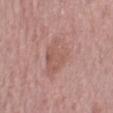The lesion was photographed on a routine skin check and not biopsied; there is no pathology result.
The subject is a female approximately 60 years of age.
The lesion is located on the left lower leg.
An algorithmic analysis of the crop reported a footprint of about 14 mm², an eccentricity of roughly 0.65, and two-axis asymmetry of about 0.3. The software also gave about 7 CIELAB-L* units darker than the surrounding skin and a lesion-to-skin contrast of about 5 (normalized; higher = more distinct).
A lesion tile, about 15 mm wide, cut from a 3D total-body photograph.
Captured under white-light illumination.